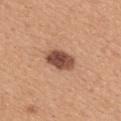{"biopsy_status": "not biopsied; imaged during a skin examination", "lesion_size": {"long_diameter_mm_approx": 4.0}, "site": "back", "image": {"source": "total-body photography crop", "field_of_view_mm": 15}, "patient": {"sex": "female", "age_approx": 30}, "lighting": "white-light"}